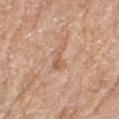Captured during whole-body skin photography for melanoma surveillance; the lesion was not biopsied. The patient is a female aged 73 to 77. A close-up tile cropped from a whole-body skin photograph, about 15 mm across. On the left thigh. The recorded lesion diameter is about 3.5 mm.